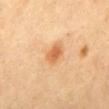  biopsy_status: not biopsied; imaged during a skin examination
  patient:
    sex: female
    age_approx: 60
  image:
    source: total-body photography crop
    field_of_view_mm: 15
  site: back